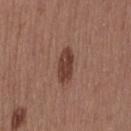* workup — catalogued during a skin exam; not biopsied
* patient — female, approximately 40 years of age
* illumination — white-light illumination
* acquisition — total-body-photography crop, ~15 mm field of view
* anatomic site — the lower back